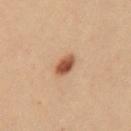{"biopsy_status": "not biopsied; imaged during a skin examination", "image": {"source": "total-body photography crop", "field_of_view_mm": 15}, "lighting": "cross-polarized", "lesion_size": {"long_diameter_mm_approx": 3.0}, "patient": {"sex": "female", "age_approx": 30}, "site": "mid back"}